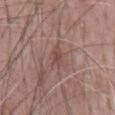No biopsy was performed on this lesion — it was imaged during a full skin examination and was not determined to be concerning.